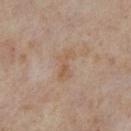The lesion was tiled from a total-body skin photograph and was not biopsied. The lesion is located on the right lower leg. A 15 mm close-up tile from a total-body photography series done for melanoma screening. A female patient in their 50s. The lesion-visualizer software estimated a lesion area of about 6.5 mm² and two-axis asymmetry of about 0.4. The analysis additionally found a mean CIELAB color near L≈53 a*≈15 b*≈29, about 5 CIELAB-L* units darker than the surrounding skin, and a lesion-to-skin contrast of about 5 (normalized; higher = more distinct). It also reported border irregularity of about 4.5 on a 0–10 scale, a within-lesion color-variation index near 3.5/10, and peripheral color asymmetry of about 1. The software also gave an automated nevus-likeness rating near 0 out of 100 and a lesion-detection confidence of about 100/100. This is a cross-polarized tile. Approximately 4 mm at its widest.